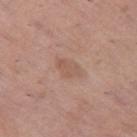Assessment:
Imaged during a routine full-body skin examination; the lesion was not biopsied and no histopathology is available.
Background:
The recorded lesion diameter is about 3.5 mm. The lesion is on the left thigh. A female patient aged approximately 65. The lesion-visualizer software estimated roughly 6 lightness units darker than nearby skin and a lesion-to-skin contrast of about 5 (normalized; higher = more distinct). A 15 mm crop from a total-body photograph taken for skin-cancer surveillance.